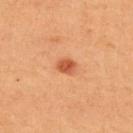notes: catalogued during a skin exam; not biopsied.